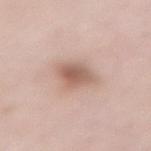No biopsy was performed on this lesion — it was imaged during a full skin examination and was not determined to be concerning. The patient is a female aged around 50. Imaged with white-light lighting. The total-body-photography lesion software estimated an area of roughly 7.5 mm² and a symmetry-axis asymmetry near 0.2. It also reported an average lesion color of about L≈59 a*≈19 b*≈26 (CIELAB). It also reported a border-irregularity index near 2/10, a within-lesion color-variation index near 4/10, and radial color variation of about 1. From the abdomen. A close-up tile cropped from a whole-body skin photograph, about 15 mm across. Longest diameter approximately 3.5 mm.